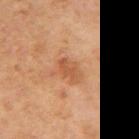Assessment:
Recorded during total-body skin imaging; not selected for excision or biopsy.
Image and clinical context:
The lesion's longest dimension is about 3 mm. A female subject, about 60 years old. Located on the right upper arm. A lesion tile, about 15 mm wide, cut from a 3D total-body photograph.